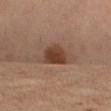| key | value |
|---|---|
| biopsy status | total-body-photography surveillance lesion; no biopsy |
| site | the leg |
| diameter | ~5 mm (longest diameter) |
| image | total-body-photography crop, ~15 mm field of view |
| patient | female, aged 63 to 67 |
| illumination | cross-polarized illumination |